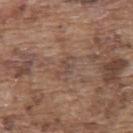biopsy_status: not biopsied; imaged during a skin examination
lighting: white-light
patient:
  sex: male
  age_approx: 75
site: back
image:
  source: total-body photography crop
  field_of_view_mm: 15
lesion_size:
  long_diameter_mm_approx: 3.0